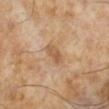The lesion was tiled from a total-body skin photograph and was not biopsied. A lesion tile, about 15 mm wide, cut from a 3D total-body photograph. The patient is a male aged around 65.